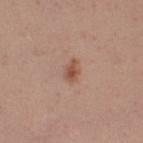  biopsy_status: not biopsied; imaged during a skin examination
  lesion_size:
    long_diameter_mm_approx: 2.5
  lighting: white-light
  image:
    source: total-body photography crop
    field_of_view_mm: 15
  automated_metrics:
    area_mm2_approx: 3.0
    eccentricity: 0.8
    shape_asymmetry: 0.3
    cielab_L: 52
    cielab_a: 22
    cielab_b: 29
    vs_skin_darker_L: 10.0
    vs_skin_contrast_norm: 7.5
    border_irregularity_0_10: 3.0
    color_variation_0_10: 2.5
    peripheral_color_asymmetry: 0.5
    nevus_likeness_0_100: 75
    lesion_detection_confidence_0_100: 100
  site: leg
  patient:
    sex: female
    age_approx: 30